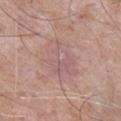Imaged during a routine full-body skin examination; the lesion was not biopsied and no histopathology is available. Located on the right lower leg. This image is a 15 mm lesion crop taken from a total-body photograph. About 5.5 mm across. A male patient aged 73 to 77.